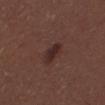<record>
  <biopsy_status>not biopsied; imaged during a skin examination</biopsy_status>
  <site>back</site>
  <patient>
    <sex>male</sex>
    <age_approx>30</age_approx>
  </patient>
  <image>
    <source>total-body photography crop</source>
    <field_of_view_mm>15</field_of_view_mm>
  </image>
</record>